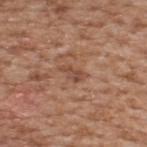{
  "biopsy_status": "not biopsied; imaged during a skin examination",
  "image": {
    "source": "total-body photography crop",
    "field_of_view_mm": 15
  },
  "site": "upper back",
  "patient": {
    "sex": "male",
    "age_approx": 65
  },
  "lesion_size": {
    "long_diameter_mm_approx": 3.0
  }
}